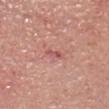This lesion was catalogued during total-body skin photography and was not selected for biopsy. A 15 mm close-up tile from a total-body photography series done for melanoma screening. From the head or neck. This is a white-light tile. Measured at roughly 2.5 mm in maximum diameter. An algorithmic analysis of the crop reported an area of roughly 2.5 mm² and two-axis asymmetry of about 0.25. And it measured a mean CIELAB color near L≈55 a*≈30 b*≈24, about 9 CIELAB-L* units darker than the surrounding skin, and a normalized lesion–skin contrast near 6. And it measured a border-irregularity rating of about 3.5/10 and a within-lesion color-variation index near 0/10. A male patient, approximately 65 years of age.